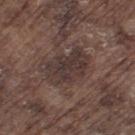Located on the left thigh. Automated image analysis of the tile measured an average lesion color of about L≈34 a*≈14 b*≈17 (CIELAB) and about 8 CIELAB-L* units darker than the surrounding skin. The analysis additionally found a border-irregularity index near 3/10 and internal color variation of about 3.5 on a 0–10 scale. The tile uses white-light illumination. About 5 mm across. Cropped from a whole-body photographic skin survey; the tile spans about 15 mm. A male subject, aged around 75.